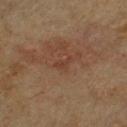No biopsy was performed on this lesion — it was imaged during a full skin examination and was not determined to be concerning. A male subject in their mid-60s. The lesion is located on the chest. Longest diameter approximately 2.5 mm. The tile uses cross-polarized illumination. A roughly 15 mm field-of-view crop from a total-body skin photograph.